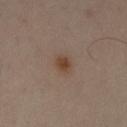Assessment: Recorded during total-body skin imaging; not selected for excision or biopsy. Image and clinical context: About 2 mm across. A male patient aged around 55. Captured under cross-polarized illumination. An algorithmic analysis of the crop reported an automated nevus-likeness rating near 95 out of 100 and a lesion-detection confidence of about 100/100. A 15 mm crop from a total-body photograph taken for skin-cancer surveillance. On the right upper arm.